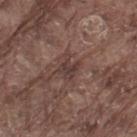Assessment:
The lesion was tiled from a total-body skin photograph and was not biopsied.
Acquisition and patient details:
A male patient, about 80 years old. On the left thigh. The recorded lesion diameter is about 2.5 mm. The tile uses white-light illumination. Cropped from a whole-body photographic skin survey; the tile spans about 15 mm.